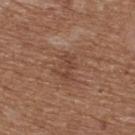| key | value |
|---|---|
| workup | catalogued during a skin exam; not biopsied |
| site | the upper back |
| lesion size | ~3 mm (longest diameter) |
| imaging modality | ~15 mm tile from a whole-body skin photo |
| subject | female, about 75 years old |
| image-analysis metrics | an average lesion color of about L≈43 a*≈21 b*≈28 (CIELAB), roughly 7 lightness units darker than nearby skin, and a normalized border contrast of about 5.5; a border-irregularity index near 6/10, a within-lesion color-variation index near 0/10, and peripheral color asymmetry of about 0 |